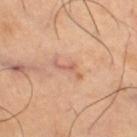Notes:
• follow-up · no biopsy performed (imaged during a skin exam)
• image source · ~15 mm tile from a whole-body skin photo
• body site · the right thigh
• subject · male, aged around 65